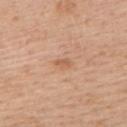biopsy_status: not biopsied; imaged during a skin examination
site: upper back
lesion_size:
  long_diameter_mm_approx: 2.0
lighting: white-light
patient:
  sex: male
  age_approx: 35
image:
  source: total-body photography crop
  field_of_view_mm: 15